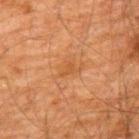This lesion was catalogued during total-body skin photography and was not selected for biopsy. An algorithmic analysis of the crop reported an average lesion color of about L≈42 a*≈21 b*≈34 (CIELAB), roughly 5 lightness units darker than nearby skin, and a lesion-to-skin contrast of about 5 (normalized; higher = more distinct). It also reported border irregularity of about 5.5 on a 0–10 scale, internal color variation of about 1 on a 0–10 scale, and a peripheral color-asymmetry measure near 0.5. And it measured a nevus-likeness score of about 0/100 and lesion-presence confidence of about 100/100. A 15 mm close-up extracted from a 3D total-body photography capture. Longest diameter approximately 3 mm. Located on the upper back. A male patient aged 58–62. Captured under cross-polarized illumination.